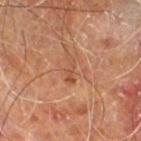Case summary:
• follow-up — total-body-photography surveillance lesion; no biopsy
• location — the right leg
• patient — male, in their 60s
• automated metrics — a mean CIELAB color near L≈49 a*≈24 b*≈33 and roughly 7 lightness units darker than nearby skin; a border-irregularity index near 6.5/10 and internal color variation of about 0 on a 0–10 scale
• image — ~15 mm tile from a whole-body skin photo
• illumination — cross-polarized